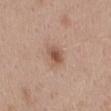Findings:
• biopsy status: catalogued during a skin exam; not biopsied
• anatomic site: the mid back
• subject: female, aged 38 to 42
• lesion size: ≈2.5 mm
• imaging modality: 15 mm crop, total-body photography